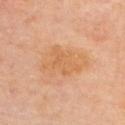  biopsy_status: not biopsied; imaged during a skin examination
  patient:
    sex: female
    age_approx: 60
  automated_metrics:
    area_mm2_approx: 14.0
    eccentricity: 0.8
    shape_asymmetry: 0.3
    cielab_L: 65
    cielab_a: 23
    cielab_b: 41
    vs_skin_darker_L: 7.0
    vs_skin_contrast_norm: 6.0
    border_irregularity_0_10: 5.0
    peripheral_color_asymmetry: 1.0
    nevus_likeness_0_100: 0
    lesion_detection_confidence_0_100: 100
  image:
    source: total-body photography crop
    field_of_view_mm: 15
  site: upper back
  lesion_size:
    long_diameter_mm_approx: 5.0